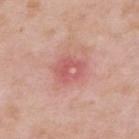Impression: This lesion was catalogued during total-body skin photography and was not selected for biopsy. Acquisition and patient details: The patient is a male in their mid-50s. On the upper back. About 3.5 mm across. A roughly 15 mm field-of-view crop from a total-body skin photograph.